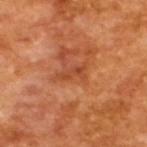Impression: No biopsy was performed on this lesion — it was imaged during a full skin examination and was not determined to be concerning. Image and clinical context: The subject is a male aged around 65. Cropped from a total-body skin-imaging series; the visible field is about 15 mm.